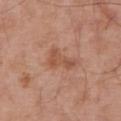Q: Was a biopsy performed?
A: no biopsy performed (imaged during a skin exam)
Q: Who is the patient?
A: male, in their mid- to late 50s
Q: How was this image acquired?
A: ~15 mm tile from a whole-body skin photo
Q: How large is the lesion?
A: ~4 mm (longest diameter)
Q: Automated lesion metrics?
A: a lesion–skin lightness drop of about 8 and a lesion-to-skin contrast of about 6 (normalized; higher = more distinct); border irregularity of about 6 on a 0–10 scale, a color-variation rating of about 2/10, and a peripheral color-asymmetry measure near 0.5
Q: How was the tile lit?
A: white-light
Q: What is the anatomic site?
A: the back